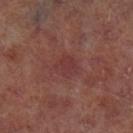Notes:
– patient — male, approximately 65 years of age
– lighting — cross-polarized illumination
– anatomic site — the left lower leg
– image — ~15 mm tile from a whole-body skin photo
– lesion diameter — about 2.5 mm